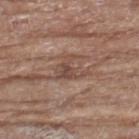No biopsy was performed on this lesion — it was imaged during a full skin examination and was not determined to be concerning. Measured at roughly 3.5 mm in maximum diameter. The subject is a female in their mid- to late 70s. Located on the left thigh. Cropped from a total-body skin-imaging series; the visible field is about 15 mm. An algorithmic analysis of the crop reported a lesion area of about 6.5 mm² and a shape eccentricity near 0.55. It also reported a color-variation rating of about 3/10 and radial color variation of about 1. It also reported a classifier nevus-likeness of about 0/100.